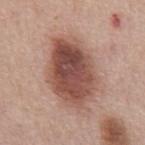<case>
  <biopsy_status>not biopsied; imaged during a skin examination</biopsy_status>
  <site>front of the torso</site>
  <lesion_size>
    <long_diameter_mm_approx>8.0</long_diameter_mm_approx>
  </lesion_size>
  <patient>
    <sex>male</sex>
    <age_approx>65</age_approx>
  </patient>
  <automated_metrics>
    <area_mm2_approx>32.0</area_mm2_approx>
    <eccentricity>0.75</eccentricity>
    <shape_asymmetry>0.1</shape_asymmetry>
    <vs_skin_contrast_norm>10.5</vs_skin_contrast_norm>
    <color_variation_0_10>7.0</color_variation_0_10>
    <peripheral_color_asymmetry>2.5</peripheral_color_asymmetry>
  </automated_metrics>
  <image>
    <source>total-body photography crop</source>
    <field_of_view_mm>15</field_of_view_mm>
  </image>
</case>